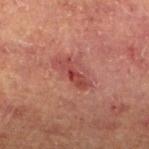  lighting: cross-polarized
  site: leg
  lesion_size:
    long_diameter_mm_approx: 4.0
  image:
    source: total-body photography crop
    field_of_view_mm: 15
  patient:
    sex: male
    age_approx: 65